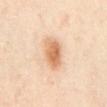Case summary:
– notes — total-body-photography surveillance lesion; no biopsy